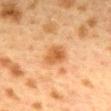Impression:
The lesion was tiled from a total-body skin photograph and was not biopsied.
Context:
Captured under cross-polarized illumination. A female subject, roughly 40 years of age. Automated tile analysis of the lesion measured two-axis asymmetry of about 0.25. It also reported a lesion color around L≈49 a*≈22 b*≈38 in CIELAB and about 10 CIELAB-L* units darker than the surrounding skin. The software also gave a classifier nevus-likeness of about 90/100. The recorded lesion diameter is about 3 mm. A lesion tile, about 15 mm wide, cut from a 3D total-body photograph. On the mid back.